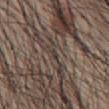Q: Was this lesion biopsied?
A: no biopsy performed (imaged during a skin exam)
Q: What is the imaging modality?
A: total-body-photography crop, ~15 mm field of view
Q: What did automated image analysis measure?
A: an area of roughly 1 mm², a shape eccentricity near 0.75, and a shape-asymmetry score of about 0.4 (0 = symmetric); an automated nevus-likeness rating near 0 out of 100 and a lesion-detection confidence of about 0/100
Q: What lighting was used for the tile?
A: white-light
Q: What is the anatomic site?
A: the abdomen
Q: What are the patient's age and sex?
A: male, aged approximately 55